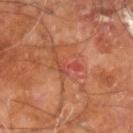Impression:
Recorded during total-body skin imaging; not selected for excision or biopsy.
Clinical summary:
Measured at roughly 3.5 mm in maximum diameter. A close-up tile cropped from a whole-body skin photograph, about 15 mm across. A male patient, about 60 years old. The total-body-photography lesion software estimated a footprint of about 3.5 mm² and a shape-asymmetry score of about 0.65 (0 = symmetric). The analysis additionally found roughly 7 lightness units darker than nearby skin and a normalized lesion–skin contrast near 5. It also reported internal color variation of about 0.5 on a 0–10 scale. On the right leg.